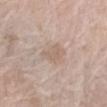The lesion was tiled from a total-body skin photograph and was not biopsied. A female subject, aged approximately 75. An algorithmic analysis of the crop reported an area of roughly 6.5 mm², a shape eccentricity near 0.85, and a shape-asymmetry score of about 0.3 (0 = symmetric). And it measured an average lesion color of about L≈62 a*≈14 b*≈25 (CIELAB) and a normalized lesion–skin contrast near 5. The software also gave a border-irregularity index near 3/10, internal color variation of about 1.5 on a 0–10 scale, and a peripheral color-asymmetry measure near 0.5. The software also gave a lesion-detection confidence of about 95/100. A lesion tile, about 15 mm wide, cut from a 3D total-body photograph. Captured under white-light illumination. Approximately 4 mm at its widest. The lesion is on the arm.